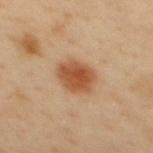Q: Was a biopsy performed?
A: imaged on a skin check; not biopsied
Q: What kind of image is this?
A: 15 mm crop, total-body photography
Q: What are the patient's age and sex?
A: male, roughly 40 years of age
Q: Lesion location?
A: the back